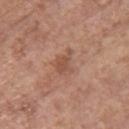Imaged during a routine full-body skin examination; the lesion was not biopsied and no histopathology is available. A region of skin cropped from a whole-body photographic capture, roughly 15 mm wide. The lesion is on the back. A female subject, about 65 years old.